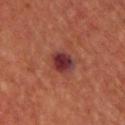Findings:
- notes · no biopsy performed (imaged during a skin exam)
- anatomic site · the chest
- lighting · cross-polarized
- subject · male, aged 63 to 67
- lesion diameter · ~3 mm (longest diameter)
- image source · ~15 mm crop, total-body skin-cancer survey